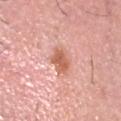Part of a total-body skin-imaging series; this lesion was reviewed on a skin check and was not flagged for biopsy.
A male patient in their 60s.
From the head or neck.
About 2.5 mm across.
The tile uses white-light illumination.
Automated image analysis of the tile measured a lesion area of about 5.5 mm² and an eccentricity of roughly 0.6. The analysis additionally found a lesion–skin lightness drop of about 12 and a normalized lesion–skin contrast near 8. And it measured a detector confidence of about 100 out of 100 that the crop contains a lesion.
This image is a 15 mm lesion crop taken from a total-body photograph.